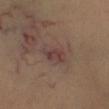Imaged with cross-polarized lighting. Located on the leg. A 15 mm close-up tile from a total-body photography series done for melanoma screening. The lesion-visualizer software estimated a mean CIELAB color near L≈40 a*≈17 b*≈20 and about 7 CIELAB-L* units darker than the surrounding skin. The lesion's longest dimension is about 4 mm. The subject is a female approximately 40 years of age.